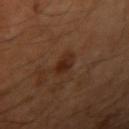No biopsy was performed on this lesion — it was imaged during a full skin examination and was not determined to be concerning.
Located on the left arm.
Captured under cross-polarized illumination.
A lesion tile, about 15 mm wide, cut from a 3D total-body photograph.
The subject is a male aged approximately 50.